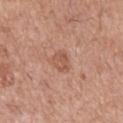notes: imaged on a skin check; not biopsied
tile lighting: white-light illumination
anatomic site: the right upper arm
image source: ~15 mm crop, total-body skin-cancer survey
patient: male, aged 53–57
lesion diameter: ~3 mm (longest diameter)